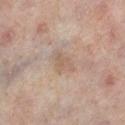notes = no biopsy performed (imaged during a skin exam); diameter = ≈3 mm; body site = the right lower leg; illumination = cross-polarized; image source = total-body-photography crop, ~15 mm field of view; subject = female, in their 50s; automated metrics = an automated nevus-likeness rating near 0 out of 100 and a detector confidence of about 100 out of 100 that the crop contains a lesion.